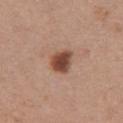notes: imaged on a skin check; not biopsied | lighting: white-light | location: the front of the torso | imaging modality: ~15 mm crop, total-body skin-cancer survey | subject: female, aged around 35 | lesion size: ~3 mm (longest diameter) | TBP lesion metrics: a footprint of about 6 mm², an eccentricity of roughly 0.55, and a shape-asymmetry score of about 0.25 (0 = symmetric); an average lesion color of about L≈46 a*≈22 b*≈28 (CIELAB), roughly 16 lightness units darker than nearby skin, and a lesion-to-skin contrast of about 11 (normalized; higher = more distinct); a border-irregularity rating of about 2.5/10, a within-lesion color-variation index near 4/10, and a peripheral color-asymmetry measure near 1; a classifier nevus-likeness of about 100/100 and a detector confidence of about 100 out of 100 that the crop contains a lesion.